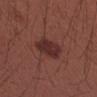Clinical impression: The lesion was tiled from a total-body skin photograph and was not biopsied.